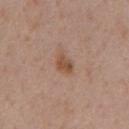Findings:
- notes: no biopsy performed (imaged during a skin exam)
- imaging modality: ~15 mm tile from a whole-body skin photo
- patient: male, aged around 60
- size: ~3 mm (longest diameter)
- body site: the chest
- image-analysis metrics: an area of roughly 3.5 mm², a shape eccentricity near 0.8, and a shape-asymmetry score of about 0.25 (0 = symmetric)
- tile lighting: white-light illumination